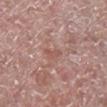biopsy status: catalogued during a skin exam; not biopsied
size: ≈3 mm
imaging modality: ~15 mm crop, total-body skin-cancer survey
anatomic site: the right lower leg
automated metrics: a footprint of about 2.5 mm² and a shape-asymmetry score of about 0.45 (0 = symmetric); an average lesion color of about L≈54 a*≈23 b*≈25 (CIELAB) and about 7 CIELAB-L* units darker than the surrounding skin; a border-irregularity rating of about 5/10, a within-lesion color-variation index near 0/10, and a peripheral color-asymmetry measure near 0
tile lighting: white-light illumination
patient: male, in their mid-60s